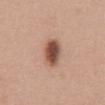Q: Is there a histopathology result?
A: imaged on a skin check; not biopsied
Q: What kind of image is this?
A: ~15 mm tile from a whole-body skin photo
Q: Who is the patient?
A: female, about 60 years old
Q: Lesion location?
A: the abdomen
Q: How large is the lesion?
A: ~4 mm (longest diameter)
Q: Automated lesion metrics?
A: an area of roughly 8.5 mm², a shape eccentricity near 0.85, and a symmetry-axis asymmetry near 0.15; a mean CIELAB color near L≈51 a*≈21 b*≈28, about 16 CIELAB-L* units darker than the surrounding skin, and a normalized border contrast of about 10.5; a border-irregularity index near 1.5/10, a color-variation rating of about 7/10, and radial color variation of about 2
Q: Illumination type?
A: white-light